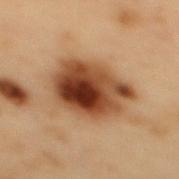– notes — no biopsy performed (imaged during a skin exam)
– diameter — ~6.5 mm (longest diameter)
– illumination — cross-polarized illumination
– image source — ~15 mm crop, total-body skin-cancer survey
– TBP lesion metrics — a lesion area of about 26 mm² and an outline eccentricity of about 0.55 (0 = round, 1 = elongated); an average lesion color of about L≈35 a*≈19 b*≈29 (CIELAB) and about 16 CIELAB-L* units darker than the surrounding skin; border irregularity of about 2 on a 0–10 scale and a peripheral color-asymmetry measure near 4; an automated nevus-likeness rating near 85 out of 100 and lesion-presence confidence of about 100/100
– patient — male, in their mid- to late 50s
– anatomic site — the mid back